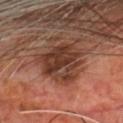No biopsy was performed on this lesion — it was imaged during a full skin examination and was not determined to be concerning.
A 15 mm close-up extracted from a 3D total-body photography capture.
A male subject approximately 70 years of age.
From the head or neck.
The lesion-visualizer software estimated a lesion area of about 19 mm², an eccentricity of roughly 0.5, and a symmetry-axis asymmetry near 0.3. The analysis additionally found an average lesion color of about L≈35 a*≈20 b*≈26 (CIELAB), roughly 11 lightness units darker than nearby skin, and a normalized lesion–skin contrast near 9.5. The analysis additionally found an automated nevus-likeness rating near 5 out of 100 and a detector confidence of about 100 out of 100 that the crop contains a lesion.